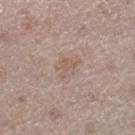location = the right lower leg | subject = female, aged 48 to 52 | acquisition = ~15 mm tile from a whole-body skin photo | diameter = ≈2.5 mm | automated lesion analysis = a footprint of about 3.5 mm² and a symmetry-axis asymmetry near 0.5; a nevus-likeness score of about 0/100 and lesion-presence confidence of about 100/100 | tile lighting = white-light illumination.